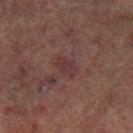Assessment:
Part of a total-body skin-imaging series; this lesion was reviewed on a skin check and was not flagged for biopsy.
Context:
Captured under cross-polarized illumination. A close-up tile cropped from a whole-body skin photograph, about 15 mm across. Measured at roughly 2.5 mm in maximum diameter. From the left lower leg. The lesion-visualizer software estimated an automated nevus-likeness rating near 0 out of 100 and a detector confidence of about 100 out of 100 that the crop contains a lesion. A male subject, in their mid-60s.